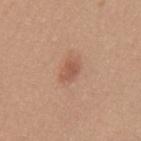| key | value |
|---|---|
| workup | total-body-photography surveillance lesion; no biopsy |
| subject | female, approximately 35 years of age |
| anatomic site | the mid back |
| lighting | white-light |
| imaging modality | ~15 mm crop, total-body skin-cancer survey |
| automated metrics | a lesion area of about 4.5 mm² and two-axis asymmetry of about 0.35 |
| size | about 3 mm |